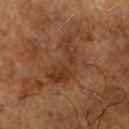Imaged during a routine full-body skin examination; the lesion was not biopsied and no histopathology is available. The subject is a male approximately 70 years of age. On the right lower leg. This image is a 15 mm lesion crop taken from a total-body photograph. The recorded lesion diameter is about 6 mm.